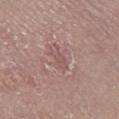Impression: Part of a total-body skin-imaging series; this lesion was reviewed on a skin check and was not flagged for biopsy. Background: The lesion is on the right lower leg. A female patient aged 18–22. A lesion tile, about 15 mm wide, cut from a 3D total-body photograph. The lesion's longest dimension is about 3 mm.